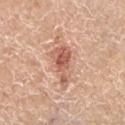No biopsy was performed on this lesion — it was imaged during a full skin examination and was not determined to be concerning. The lesion is on the left lower leg. A female patient, aged around 70. The recorded lesion diameter is about 5 mm. The tile uses white-light illumination. A 15 mm crop from a total-body photograph taken for skin-cancer surveillance.